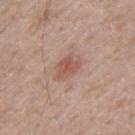The lesion-visualizer software estimated a mean CIELAB color near L≈54 a*≈23 b*≈26, about 9 CIELAB-L* units darker than the surrounding skin, and a normalized lesion–skin contrast near 6.5. A 15 mm close-up extracted from a 3D total-body photography capture. From the mid back. Imaged with white-light lighting. The recorded lesion diameter is about 3 mm. A male subject, approximately 40 years of age.